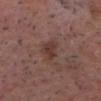A close-up tile cropped from a whole-body skin photograph, about 15 mm across.
On the head or neck.
Measured at roughly 3 mm in maximum diameter.
This is a white-light tile.
The lesion-visualizer software estimated an area of roughly 5.5 mm², an eccentricity of roughly 0.7, and a shape-asymmetry score of about 0.25 (0 = symmetric). The software also gave a lesion-to-skin contrast of about 7 (normalized; higher = more distinct). The analysis additionally found a border-irregularity rating of about 2.5/10, a within-lesion color-variation index near 2.5/10, and peripheral color asymmetry of about 1. And it measured a nevus-likeness score of about 20/100.
A male subject, aged 53–57.